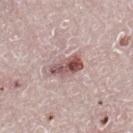Notes:
– follow-up · imaged on a skin check; not biopsied
– TBP lesion metrics · an average lesion color of about L≈54 a*≈22 b*≈19 (CIELAB) and about 15 CIELAB-L* units darker than the surrounding skin; an automated nevus-likeness rating near 55 out of 100
– acquisition · ~15 mm crop, total-body skin-cancer survey
– lesion diameter · about 4 mm
– patient · male, approximately 65 years of age
– site · the right lower leg
– lighting · white-light